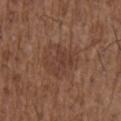Assessment: The lesion was tiled from a total-body skin photograph and was not biopsied. Context: A male subject approximately 50 years of age. Longest diameter approximately 5 mm. The tile uses white-light illumination. From the right upper arm. A close-up tile cropped from a whole-body skin photograph, about 15 mm across. An algorithmic analysis of the crop reported a lesion area of about 16 mm² and a shape-asymmetry score of about 0.2 (0 = symmetric). It also reported a nevus-likeness score of about 0/100.